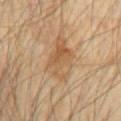Impression: Part of a total-body skin-imaging series; this lesion was reviewed on a skin check and was not flagged for biopsy. Acquisition and patient details: The lesion is located on the chest. Imaged with cross-polarized lighting. Longest diameter approximately 5 mm. The patient is a male roughly 70 years of age. A 15 mm crop from a total-body photograph taken for skin-cancer surveillance. Automated image analysis of the tile measured an area of roughly 14 mm² and a shape eccentricity near 0.8. The software also gave a lesion–skin lightness drop of about 8 and a lesion-to-skin contrast of about 6 (normalized; higher = more distinct).